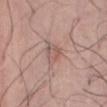Q: Was a biopsy performed?
A: imaged on a skin check; not biopsied
Q: What did automated image analysis measure?
A: a border-irregularity index near 3/10, internal color variation of about 4 on a 0–10 scale, and a peripheral color-asymmetry measure near 1.5
Q: How large is the lesion?
A: ~3 mm (longest diameter)
Q: What is the imaging modality?
A: total-body-photography crop, ~15 mm field of view
Q: What lighting was used for the tile?
A: white-light
Q: Who is the patient?
A: male, approximately 20 years of age
Q: What is the anatomic site?
A: the abdomen